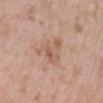Q: Is there a histopathology result?
A: total-body-photography surveillance lesion; no biopsy
Q: What is the lesion's diameter?
A: about 4.5 mm
Q: What is the anatomic site?
A: the right lower leg
Q: What did automated image analysis measure?
A: a lesion–skin lightness drop of about 8 and a normalized lesion–skin contrast near 5.5; internal color variation of about 4.5 on a 0–10 scale and radial color variation of about 1.5
Q: Patient demographics?
A: female, aged 68–72
Q: How was the tile lit?
A: white-light illumination
Q: What is the imaging modality?
A: 15 mm crop, total-body photography